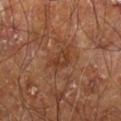biopsy_status: not biopsied; imaged during a skin examination
site: leg
lesion_size:
  long_diameter_mm_approx: 3.0
lighting: cross-polarized
patient:
  sex: male
  age_approx: 60
automated_metrics:
  cielab_L: 37
  cielab_a: 22
  cielab_b: 30
  vs_skin_darker_L: 6.0
  vs_skin_contrast_norm: 6.0
  color_variation_0_10: 3.5
image:
  source: total-body photography crop
  field_of_view_mm: 15